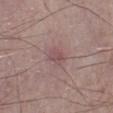<case>
  <biopsy_status>not biopsied; imaged during a skin examination</biopsy_status>
  <image>
    <source>total-body photography crop</source>
    <field_of_view_mm>15</field_of_view_mm>
  </image>
  <automated_metrics>
    <area_mm2_approx>3.0</area_mm2_approx>
    <shape_asymmetry>0.25</shape_asymmetry>
    <cielab_L>49</cielab_L>
    <cielab_a>20</cielab_a>
    <cielab_b>18</cielab_b>
    <vs_skin_darker_L>7.0</vs_skin_darker_L>
    <vs_skin_contrast_norm>5.5</vs_skin_contrast_norm>
    <border_irregularity_0_10>2.5</border_irregularity_0_10>
    <color_variation_0_10>2.5</color_variation_0_10>
    <peripheral_color_asymmetry>1.0</peripheral_color_asymmetry>
  </automated_metrics>
  <site>left lower leg</site>
  <patient>
    <sex>male</sex>
    <age_approx>75</age_approx>
  </patient>
</case>